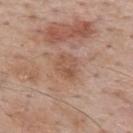Recorded during total-body skin imaging; not selected for excision or biopsy.
Cropped from a whole-body photographic skin survey; the tile spans about 15 mm.
From the upper back.
A male subject aged around 60.